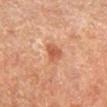- follow-up · no biopsy performed (imaged during a skin exam)
- diameter · about 2.5 mm
- anatomic site · the right lower leg
- patient · male, aged approximately 65
- lighting · cross-polarized
- image · ~15 mm crop, total-body skin-cancer survey